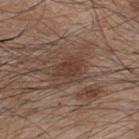Impression:
No biopsy was performed on this lesion — it was imaged during a full skin examination and was not determined to be concerning.
Context:
On the upper back. A 15 mm crop from a total-body photograph taken for skin-cancer surveillance. The patient is a male aged approximately 45.